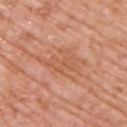Assessment:
Recorded during total-body skin imaging; not selected for excision or biopsy.